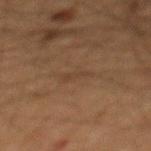Q: Was a biopsy performed?
A: imaged on a skin check; not biopsied
Q: Patient demographics?
A: male, roughly 65 years of age
Q: What is the lesion's diameter?
A: ~3 mm (longest diameter)
Q: What lighting was used for the tile?
A: cross-polarized illumination
Q: Lesion location?
A: the mid back
Q: Automated lesion metrics?
A: a lesion color around L≈32 a*≈14 b*≈25 in CIELAB, roughly 4 lightness units darker than nearby skin, and a normalized border contrast of about 4.5; a color-variation rating of about 0/10 and a peripheral color-asymmetry measure near 0; a detector confidence of about 55 out of 100 that the crop contains a lesion
Q: What is the imaging modality?
A: 15 mm crop, total-body photography